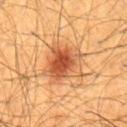Imaged during a routine full-body skin examination; the lesion was not biopsied and no histopathology is available. A male patient, in their 60s. The lesion-visualizer software estimated a lesion color around L≈53 a*≈26 b*≈39 in CIELAB and a lesion-to-skin contrast of about 8.5 (normalized; higher = more distinct). The software also gave internal color variation of about 7.5 on a 0–10 scale and a peripheral color-asymmetry measure near 2. And it measured an automated nevus-likeness rating near 100 out of 100 and a lesion-detection confidence of about 100/100. The lesion is on the chest. Cropped from a whole-body photographic skin survey; the tile spans about 15 mm.